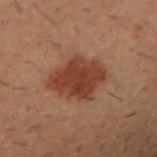subject: male, aged around 30 | site: the arm | acquisition: total-body-photography crop, ~15 mm field of view.